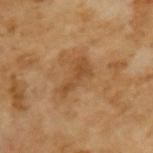Imaged during a routine full-body skin examination; the lesion was not biopsied and no histopathology is available.
A close-up tile cropped from a whole-body skin photograph, about 15 mm across.
A male patient, roughly 65 years of age.